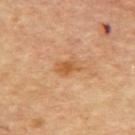<record>
  <biopsy_status>not biopsied; imaged during a skin examination</biopsy_status>
  <lesion_size>
    <long_diameter_mm_approx>3.0</long_diameter_mm_approx>
  </lesion_size>
  <image>
    <source>total-body photography crop</source>
    <field_of_view_mm>15</field_of_view_mm>
  </image>
  <patient>
    <sex>male</sex>
    <age_approx>85+</age_approx>
  </patient>
  <automated_metrics>
    <area_mm2_approx>4.5</area_mm2_approx>
    <eccentricity>0.8</eccentricity>
    <shape_asymmetry>0.3</shape_asymmetry>
    <color_variation_0_10>2.5</color_variation_0_10>
    <peripheral_color_asymmetry>0.5</peripheral_color_asymmetry>
  </automated_metrics>
  <site>upper back</site>
  <lighting>cross-polarized</lighting>
</record>